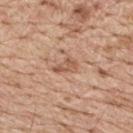Part of a total-body skin-imaging series; this lesion was reviewed on a skin check and was not flagged for biopsy. The tile uses white-light illumination. Cropped from a total-body skin-imaging series; the visible field is about 15 mm. The recorded lesion diameter is about 3 mm. A male patient aged 68 to 72. From the back.